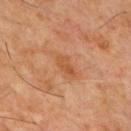Assessment:
The lesion was photographed on a routine skin check and not biopsied; there is no pathology result.
Background:
Automated tile analysis of the lesion measured an eccentricity of roughly 0.9 and a shape-asymmetry score of about 0.25 (0 = symmetric). The analysis additionally found a mean CIELAB color near L≈49 a*≈24 b*≈37, a lesion–skin lightness drop of about 7, and a lesion-to-skin contrast of about 6 (normalized; higher = more distinct). The recorded lesion diameter is about 3 mm. A 15 mm close-up extracted from a 3D total-body photography capture. From the chest. The tile uses cross-polarized illumination. A male subject in their 60s.